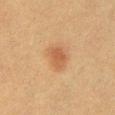workup: no biopsy performed (imaged during a skin exam)
illumination: cross-polarized illumination
site: the front of the torso
automated lesion analysis: a footprint of about 5.5 mm² and an outline eccentricity of about 0.65 (0 = round, 1 = elongated); a border-irregularity rating of about 2.5/10, a within-lesion color-variation index near 2/10, and radial color variation of about 0.5; a classifier nevus-likeness of about 95/100 and a lesion-detection confidence of about 100/100
subject: female, aged 38–42
diameter: about 3 mm
image: ~15 mm tile from a whole-body skin photo